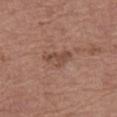follow-up = catalogued during a skin exam; not biopsied | patient = female, aged 63–67 | automated metrics = a footprint of about 6.5 mm², a shape eccentricity near 0.85, and two-axis asymmetry of about 0.4; a mean CIELAB color near L≈48 a*≈20 b*≈27, roughly 8 lightness units darker than nearby skin, and a normalized border contrast of about 6; border irregularity of about 4 on a 0–10 scale, a color-variation rating of about 2.5/10, and a peripheral color-asymmetry measure near 1 | lesion diameter = about 4 mm | lighting = white-light | location = the left thigh | image = ~15 mm crop, total-body skin-cancer survey.